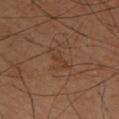lesion size: ~3.5 mm (longest diameter)
image: ~15 mm crop, total-body skin-cancer survey
patient: male, about 60 years old
lighting: cross-polarized
image-analysis metrics: a footprint of about 3.5 mm², a shape eccentricity near 0.95, and a shape-asymmetry score of about 0.5 (0 = symmetric); roughly 5 lightness units darker than nearby skin and a normalized lesion–skin contrast near 5; a border-irregularity rating of about 5.5/10, a color-variation rating of about 0.5/10, and radial color variation of about 0; an automated nevus-likeness rating near 0 out of 100 and a lesion-detection confidence of about 100/100
site: the upper back